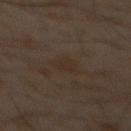follow-up: total-body-photography surveillance lesion; no biopsy
location: the abdomen
diameter: about 2.5 mm
patient: male, aged approximately 60
image-analysis metrics: a lesion–skin lightness drop of about 3 and a normalized lesion–skin contrast near 5
illumination: cross-polarized
image: ~15 mm tile from a whole-body skin photo